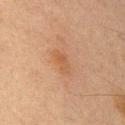* notes: no biopsy performed (imaged during a skin exam)
* illumination: cross-polarized
* automated lesion analysis: an area of roughly 3 mm², a shape eccentricity near 0.85, and two-axis asymmetry of about 0.35; about 6 CIELAB-L* units darker than the surrounding skin and a normalized border contrast of about 5.5
* acquisition: 15 mm crop, total-body photography
* subject: male, in their mid-60s
* body site: the right upper arm